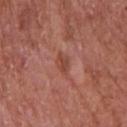image source = ~15 mm tile from a whole-body skin photo | patient = male, approximately 65 years of age | body site = the chest | lesion size = about 2.5 mm | image-analysis metrics = a lesion area of about 3.5 mm²; a lesion-detection confidence of about 100/100 | tile lighting = white-light illumination.